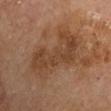<case>
  <biopsy_status>not biopsied; imaged during a skin examination</biopsy_status>
  <site>arm</site>
  <image>
    <source>total-body photography crop</source>
    <field_of_view_mm>15</field_of_view_mm>
  </image>
  <patient>
    <sex>male</sex>
    <age_approx>70</age_approx>
  </patient>
</case>